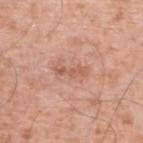follow-up — imaged on a skin check; not biopsied | image source — 15 mm crop, total-body photography | location — the left lower leg | subject — male, about 80 years old | lesion diameter — about 3.5 mm | lighting — white-light illumination | image-analysis metrics — a lesion area of about 4 mm² and an outline eccentricity of about 0.95 (0 = round, 1 = elongated); a lesion color around L≈58 a*≈24 b*≈31 in CIELAB and a normalized border contrast of about 6; border irregularity of about 4.5 on a 0–10 scale, internal color variation of about 1.5 on a 0–10 scale, and radial color variation of about 0; a nevus-likeness score of about 0/100 and a lesion-detection confidence of about 100/100.